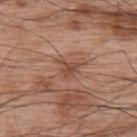Imaged during a routine full-body skin examination; the lesion was not biopsied and no histopathology is available. The tile uses white-light illumination. The lesion is located on the upper back. The lesion's longest dimension is about 2.5 mm. Cropped from a whole-body photographic skin survey; the tile spans about 15 mm. The lesion-visualizer software estimated a footprint of about 4 mm², a shape eccentricity near 0.55, and two-axis asymmetry of about 0.4. The analysis additionally found a mean CIELAB color near L≈48 a*≈22 b*≈29, roughly 8 lightness units darker than nearby skin, and a normalized lesion–skin contrast near 6.5. The patient is a male approximately 70 years of age.